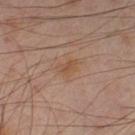<lesion>
<biopsy_status>not biopsied; imaged during a skin examination</biopsy_status>
<site>left lower leg</site>
<patient>
  <sex>male</sex>
  <age_approx>55</age_approx>
</patient>
<image>
  <source>total-body photography crop</source>
  <field_of_view_mm>15</field_of_view_mm>
</image>
<lesion_size>
  <long_diameter_mm_approx>3.0</long_diameter_mm_approx>
</lesion_size>
<automated_metrics>
  <area_mm2_approx>4.0</area_mm2_approx>
  <eccentricity>0.75</eccentricity>
  <shape_asymmetry>0.3</shape_asymmetry>
</automated_metrics>
<lighting>cross-polarized</lighting>
</lesion>